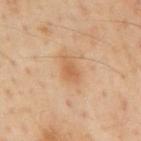On the mid back. Automated tile analysis of the lesion measured a lesion area of about 5 mm². The analysis additionally found an average lesion color of about L≈61 a*≈22 b*≈37 (CIELAB), about 8 CIELAB-L* units darker than the surrounding skin, and a normalized lesion–skin contrast near 6. And it measured a border-irregularity rating of about 2.5/10 and radial color variation of about 1. And it measured an automated nevus-likeness rating near 40 out of 100 and a detector confidence of about 100 out of 100 that the crop contains a lesion. This image is a 15 mm lesion crop taken from a total-body photograph. The subject is a male aged 53 to 57. This is a cross-polarized tile. The recorded lesion diameter is about 3 mm.